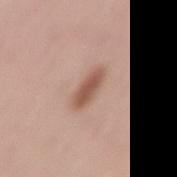Case summary:
- notes · catalogued during a skin exam; not biopsied
- size · ≈4 mm
- subject · female, aged 38–42
- location · the left thigh
- imaging modality · ~15 mm crop, total-body skin-cancer survey
- tile lighting · white-light illumination